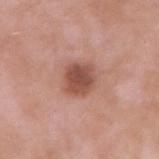Located on the arm. Cropped from a total-body skin-imaging series; the visible field is about 15 mm. Captured under white-light illumination. A male patient, approximately 55 years of age.